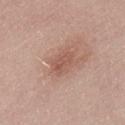A 15 mm close-up tile from a total-body photography series done for melanoma screening. On the lower back. The subject is a male aged 48 to 52.